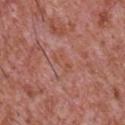• notes: imaged on a skin check; not biopsied
• anatomic site: the chest
• patient: male, approximately 45 years of age
• imaging modality: ~15 mm tile from a whole-body skin photo